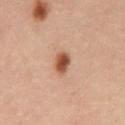Part of a total-body skin-imaging series; this lesion was reviewed on a skin check and was not flagged for biopsy. The patient is a male approximately 40 years of age. The lesion is located on the abdomen. This is a cross-polarized tile. The lesion's longest dimension is about 2.5 mm. A roughly 15 mm field-of-view crop from a total-body skin photograph.